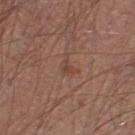workup: imaged on a skin check; not biopsied
diameter: ~3 mm (longest diameter)
anatomic site: the leg
tile lighting: white-light illumination
patient: male, in their mid- to late 50s
acquisition: 15 mm crop, total-body photography